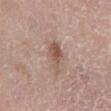biopsy status — no biopsy performed (imaged during a skin exam)
anatomic site — the left lower leg
subject — female, aged 63 to 67
lighting — white-light
TBP lesion metrics — a mean CIELAB color near L≈54 a*≈18 b*≈25 and about 10 CIELAB-L* units darker than the surrounding skin; internal color variation of about 4 on a 0–10 scale and radial color variation of about 1.5
acquisition — total-body-photography crop, ~15 mm field of view
size — ~3.5 mm (longest diameter)